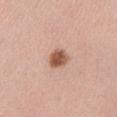Recorded during total-body skin imaging; not selected for excision or biopsy. The recorded lesion diameter is about 2.5 mm. On the arm. A region of skin cropped from a whole-body photographic capture, roughly 15 mm wide. Automated tile analysis of the lesion measured an area of roughly 5 mm², an eccentricity of roughly 0.55, and two-axis asymmetry of about 0.2. It also reported an average lesion color of about L≈55 a*≈23 b*≈30 (CIELAB), roughly 16 lightness units darker than nearby skin, and a lesion-to-skin contrast of about 10.5 (normalized; higher = more distinct). The software also gave border irregularity of about 1.5 on a 0–10 scale and peripheral color asymmetry of about 1. The analysis additionally found a classifier nevus-likeness of about 100/100 and a lesion-detection confidence of about 100/100. A female subject, aged around 35.